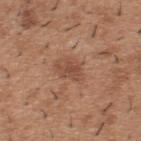notes=no biopsy performed (imaged during a skin exam)
imaging modality=15 mm crop, total-body photography
body site=the upper back
patient=male, in their 40s